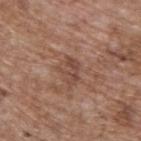workup = catalogued during a skin exam; not biopsied | lesion size = ~3 mm (longest diameter) | image source = ~15 mm tile from a whole-body skin photo | tile lighting = white-light illumination | subject = male, aged approximately 70 | automated metrics = a classifier nevus-likeness of about 0/100 and lesion-presence confidence of about 95/100 | anatomic site = the upper back.